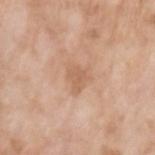Findings:
– follow-up — imaged on a skin check; not biopsied
– subject — female, about 75 years old
– lighting — white-light
– site — the left upper arm
– image source — 15 mm crop, total-body photography